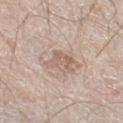Imaged during a routine full-body skin examination; the lesion was not biopsied and no histopathology is available. Automated image analysis of the tile measured an average lesion color of about L≈61 a*≈15 b*≈26 (CIELAB). It also reported an automated nevus-likeness rating near 0 out of 100 and a lesion-detection confidence of about 95/100. The lesion is located on the right thigh. The recorded lesion diameter is about 3 mm. A male subject, roughly 60 years of age. Imaged with white-light lighting. A roughly 15 mm field-of-view crop from a total-body skin photograph.